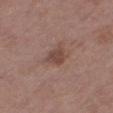biopsy status: total-body-photography surveillance lesion; no biopsy
patient: male, aged approximately 75
tile lighting: white-light illumination
image source: 15 mm crop, total-body photography
site: the right thigh